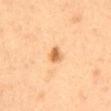Findings:
* workup · catalogued during a skin exam; not biopsied
* image-analysis metrics · an outline eccentricity of about 0.8 (0 = round, 1 = elongated) and two-axis asymmetry of about 0.3; border irregularity of about 3 on a 0–10 scale, a color-variation rating of about 3/10, and peripheral color asymmetry of about 1; an automated nevus-likeness rating near 95 out of 100
* patient · male, aged around 50
* image source · ~15 mm crop, total-body skin-cancer survey
* body site · the back
* size · about 2.5 mm